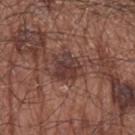Findings:
* workup: imaged on a skin check; not biopsied
* body site: the left upper arm
* automated lesion analysis: a lesion area of about 8.5 mm², an eccentricity of roughly 0.2, and a shape-asymmetry score of about 0.3 (0 = symmetric); a lesion color around L≈37 a*≈19 b*≈21 in CIELAB, a lesion–skin lightness drop of about 9, and a normalized lesion–skin contrast near 8; a nevus-likeness score of about 0/100 and lesion-presence confidence of about 95/100
* diameter: ≈3.5 mm
* acquisition: 15 mm crop, total-body photography
* subject: male, roughly 65 years of age